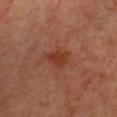Case summary:
• notes: no biopsy performed (imaged during a skin exam)
• image: ~15 mm tile from a whole-body skin photo
• lesion size: ≈3 mm
• subject: female, approximately 40 years of age
• tile lighting: cross-polarized
• location: the chest